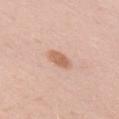Captured during whole-body skin photography for melanoma surveillance; the lesion was not biopsied. Cropped from a whole-body photographic skin survey; the tile spans about 15 mm. The tile uses white-light illumination. A female subject, aged around 30. Automated tile analysis of the lesion measured an average lesion color of about L≈63 a*≈22 b*≈31 (CIELAB), a lesion–skin lightness drop of about 11, and a normalized lesion–skin contrast near 7.5. And it measured a border-irregularity rating of about 2/10, a within-lesion color-variation index near 2.5/10, and a peripheral color-asymmetry measure near 1. Approximately 2.5 mm at its widest. On the right upper arm.